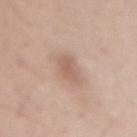Case summary:
– biopsy status: total-body-photography surveillance lesion; no biopsy
– diameter: about 3 mm
– patient: male, about 30 years old
– body site: the mid back
– acquisition: ~15 mm tile from a whole-body skin photo